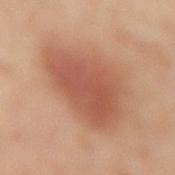Clinical impression:
Captured during whole-body skin photography for melanoma surveillance; the lesion was not biopsied.
Image and clinical context:
A female subject approximately 55 years of age. Imaged with cross-polarized lighting. This image is a 15 mm lesion crop taken from a total-body photograph. Located on the lower back.